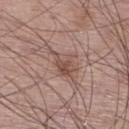Impression:
No biopsy was performed on this lesion — it was imaged during a full skin examination and was not determined to be concerning.
Context:
On the right lower leg. The tile uses white-light illumination. A male subject about 60 years old. A close-up tile cropped from a whole-body skin photograph, about 15 mm across.